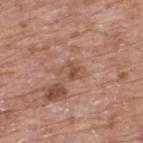Assessment:
Recorded during total-body skin imaging; not selected for excision or biopsy.
Clinical summary:
A male patient, aged 63 to 67. Cropped from a total-body skin-imaging series; the visible field is about 15 mm. From the upper back.